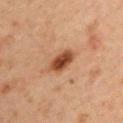Q: What is the lesion's diameter?
A: about 3.5 mm
Q: Illumination type?
A: cross-polarized
Q: Automated lesion metrics?
A: a lesion area of about 6.5 mm², a shape eccentricity near 0.75, and a shape-asymmetry score of about 0.25 (0 = symmetric); a mean CIELAB color near L≈36 a*≈20 b*≈28 and a lesion-to-skin contrast of about 10.5 (normalized; higher = more distinct); lesion-presence confidence of about 100/100
Q: What is the imaging modality?
A: ~15 mm tile from a whole-body skin photo
Q: Lesion location?
A: the right upper arm
Q: Patient demographics?
A: male, approximately 60 years of age